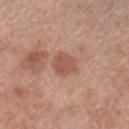workup=no biopsy performed (imaged during a skin exam) | acquisition=total-body-photography crop, ~15 mm field of view | lesion diameter=about 3 mm | anatomic site=the left lower leg | subject=male, aged 68 to 72 | lighting=white-light.